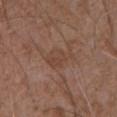Recorded during total-body skin imaging; not selected for excision or biopsy. A 15 mm close-up extracted from a 3D total-body photography capture. The lesion is on the abdomen. A male subject aged 73 to 77.